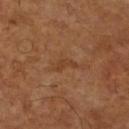• notes: imaged on a skin check; not biopsied
• TBP lesion metrics: about 6 CIELAB-L* units darker than the surrounding skin and a normalized lesion–skin contrast near 5.5
• acquisition: 15 mm crop, total-body photography
• diameter: ~2.5 mm (longest diameter)
• lighting: cross-polarized
• location: the right lower leg
• patient: male, approximately 60 years of age